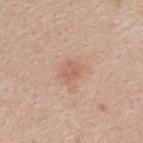Located on the upper back. Imaged with white-light lighting. A female subject, in their mid- to late 50s. An algorithmic analysis of the crop reported an area of roughly 4.5 mm² and two-axis asymmetry of about 0.25. It also reported a lesion color around L≈61 a*≈22 b*≈29 in CIELAB, a lesion–skin lightness drop of about 7, and a normalized border contrast of about 5. And it measured a border-irregularity index near 2.5/10, internal color variation of about 2.5 on a 0–10 scale, and a peripheral color-asymmetry measure near 1. The software also gave an automated nevus-likeness rating near 5 out of 100 and a lesion-detection confidence of about 100/100. This image is a 15 mm lesion crop taken from a total-body photograph.